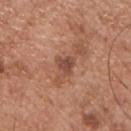The lesion was tiled from a total-body skin photograph and was not biopsied. From the chest. Automated tile analysis of the lesion measured a classifier nevus-likeness of about 0/100 and lesion-presence confidence of about 100/100. Captured under white-light illumination. A 15 mm crop from a total-body photograph taken for skin-cancer surveillance. A male patient, roughly 55 years of age.